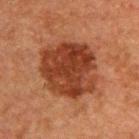Impression:
The lesion was photographed on a routine skin check and not biopsied; there is no pathology result.
Acquisition and patient details:
A male patient in their 50s. A 15 mm close-up tile from a total-body photography series done for melanoma screening. On the upper back.